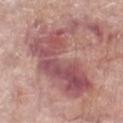Part of a total-body skin-imaging series; this lesion was reviewed on a skin check and was not flagged for biopsy. The lesion is located on the leg. A female patient roughly 60 years of age. The tile uses white-light illumination. About 9 mm across. A 15 mm crop from a total-body photograph taken for skin-cancer surveillance.